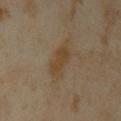| feature | finding |
|---|---|
| notes | total-body-photography surveillance lesion; no biopsy |
| lesion size | about 5 mm |
| imaging modality | 15 mm crop, total-body photography |
| body site | the left upper arm |
| subject | female, aged around 35 |